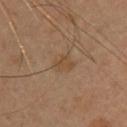| key | value |
|---|---|
| lesion size | ~2.5 mm (longest diameter) |
| image | 15 mm crop, total-body photography |
| TBP lesion metrics | a lesion area of about 4 mm² and an eccentricity of roughly 0.65; an automated nevus-likeness rating near 0 out of 100 and a detector confidence of about 100 out of 100 that the crop contains a lesion |
| subject | female, approximately 35 years of age |
| anatomic site | the head or neck |